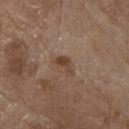Automated tile analysis of the lesion measured an average lesion color of about L≈42 a*≈17 b*≈28 (CIELAB), a lesion–skin lightness drop of about 8, and a normalized border contrast of about 7.5. The software also gave a border-irregularity index near 4/10, internal color variation of about 1 on a 0–10 scale, and radial color variation of about 0.5. It also reported a nevus-likeness score of about 10/100 and lesion-presence confidence of about 100/100. About 3 mm across. The lesion is located on the chest. A male patient, roughly 80 years of age. A close-up tile cropped from a whole-body skin photograph, about 15 mm across.